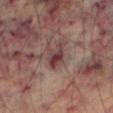Imaged with cross-polarized lighting. A male subject roughly 70 years of age. From the leg. A 15 mm crop from a total-body photograph taken for skin-cancer surveillance. The lesion-visualizer software estimated a lesion color around L≈37 a*≈21 b*≈17 in CIELAB, about 11 CIELAB-L* units darker than the surrounding skin, and a lesion-to-skin contrast of about 10 (normalized; higher = more distinct). About 3.5 mm across.